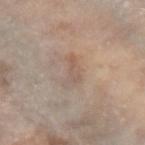workup = no biopsy performed (imaged during a skin exam)
subject = female, approximately 70 years of age
tile lighting = white-light illumination
acquisition = ~15 mm tile from a whole-body skin photo
location = the right forearm
lesion diameter = about 4 mm